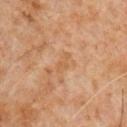Recorded during total-body skin imaging; not selected for excision or biopsy.
A male subject roughly 60 years of age.
A roughly 15 mm field-of-view crop from a total-body skin photograph.
The tile uses cross-polarized illumination.
Located on the front of the torso.